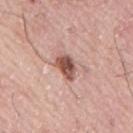Clinical impression:
No biopsy was performed on this lesion — it was imaged during a full skin examination and was not determined to be concerning.
Context:
The patient is a male in their mid-70s. This image is a 15 mm lesion crop taken from a total-body photograph. Located on the mid back.